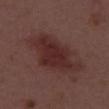follow-up = imaged on a skin check; not biopsied | diameter = ~8 mm (longest diameter) | tile lighting = white-light illumination | patient = male, approximately 55 years of age | image = 15 mm crop, total-body photography | TBP lesion metrics = a lesion area of about 22 mm² and a shape eccentricity near 0.85; roughly 8 lightness units darker than nearby skin; a lesion-detection confidence of about 100/100 | location = the upper back.